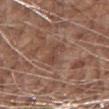Q: Is there a histopathology result?
A: catalogued during a skin exam; not biopsied
Q: What is the imaging modality?
A: total-body-photography crop, ~15 mm field of view
Q: Where on the body is the lesion?
A: the right forearm
Q: Illumination type?
A: white-light
Q: Patient demographics?
A: male, in their 70s